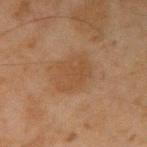{"biopsy_status": "not biopsied; imaged during a skin examination", "image": {"source": "total-body photography crop", "field_of_view_mm": 15}, "patient": {"sex": "male", "age_approx": 45}, "site": "right upper arm"}